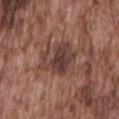{"biopsy_status": "not biopsied; imaged during a skin examination", "patient": {"sex": "male", "age_approx": 75}, "lesion_size": {"long_diameter_mm_approx": 6.0}, "automated_metrics": {"vs_skin_darker_L": 9.0, "vs_skin_contrast_norm": 8.0, "border_irregularity_0_10": 5.0, "peripheral_color_asymmetry": 1.5, "nevus_likeness_0_100": 0, "lesion_detection_confidence_0_100": 95}, "lighting": "white-light", "image": {"source": "total-body photography crop", "field_of_view_mm": 15}, "site": "mid back"}